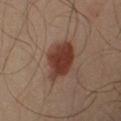workup: catalogued during a skin exam; not biopsied | body site: the right upper arm | acquisition: ~15 mm crop, total-body skin-cancer survey | subject: male, aged approximately 65.